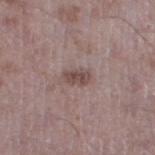Longest diameter approximately 2.5 mm.
Located on the left thigh.
The patient is a male aged approximately 50.
Automated image analysis of the tile measured a border-irregularity rating of about 2/10 and internal color variation of about 2.5 on a 0–10 scale.
A close-up tile cropped from a whole-body skin photograph, about 15 mm across.
Captured under white-light illumination.